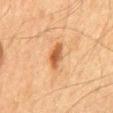Cropped from a total-body skin-imaging series; the visible field is about 15 mm. Located on the back. Automated tile analysis of the lesion measured a footprint of about 4.5 mm², a shape eccentricity near 0.85, and a symmetry-axis asymmetry near 0.3. And it measured a nevus-likeness score of about 90/100 and lesion-presence confidence of about 100/100. Measured at roughly 3.5 mm in maximum diameter. The subject is a male about 65 years old.